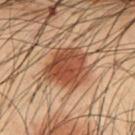The lesion was photographed on a routine skin check and not biopsied; there is no pathology result. On the abdomen. Captured under cross-polarized illumination. The recorded lesion diameter is about 5.5 mm. The total-body-photography lesion software estimated a footprint of about 19 mm² and a shape-asymmetry score of about 0.25 (0 = symmetric). A male subject about 55 years old. Cropped from a total-body skin-imaging series; the visible field is about 15 mm.